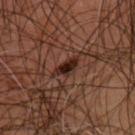The lesion is on the upper back. A 15 mm close-up extracted from a 3D total-body photography capture. Approximately 3.5 mm at its widest. A male patient aged around 45. Imaged with cross-polarized lighting. An algorithmic analysis of the crop reported an area of roughly 5 mm², an outline eccentricity of about 0.85 (0 = round, 1 = elongated), and a shape-asymmetry score of about 0.4 (0 = symmetric). It also reported a mean CIELAB color near L≈24 a*≈19 b*≈21, a lesion–skin lightness drop of about 11, and a normalized lesion–skin contrast near 11.5.